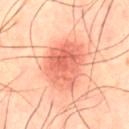{
  "biopsy_status": "not biopsied; imaged during a skin examination",
  "image": {
    "source": "total-body photography crop",
    "field_of_view_mm": 15
  },
  "lesion_size": {
    "long_diameter_mm_approx": 6.0
  },
  "site": "abdomen",
  "lighting": "cross-polarized",
  "patient": {
    "sex": "male",
    "age_approx": 50
  }
}